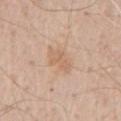  biopsy_status: not biopsied; imaged during a skin examination
  automated_metrics:
    cielab_L: 64
    cielab_a: 18
    cielab_b: 32
    nevus_likeness_0_100: 0
  image:
    source: total-body photography crop
    field_of_view_mm: 15
  site: front of the torso
  lesion_size:
    long_diameter_mm_approx: 4.0
  patient:
    sex: male
    age_approx: 70